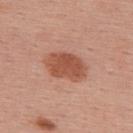Part of a total-body skin-imaging series; this lesion was reviewed on a skin check and was not flagged for biopsy. The total-body-photography lesion software estimated an average lesion color of about L≈53 a*≈26 b*≈32 (CIELAB), about 12 CIELAB-L* units darker than the surrounding skin, and a lesion-to-skin contrast of about 8.5 (normalized; higher = more distinct). And it measured border irregularity of about 2 on a 0–10 scale, a within-lesion color-variation index near 3/10, and radial color variation of about 1. The software also gave an automated nevus-likeness rating near 95 out of 100 and a lesion-detection confidence of about 100/100. The tile uses white-light illumination. Longest diameter approximately 5.5 mm. Cropped from a whole-body photographic skin survey; the tile spans about 15 mm. The subject is a female in their mid- to late 50s. On the upper back.